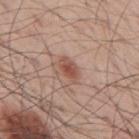The lesion is on the back.
The lesion-visualizer software estimated a mean CIELAB color near L≈52 a*≈22 b*≈28, roughly 11 lightness units darker than nearby skin, and a normalized lesion–skin contrast near 8.
The patient is a male aged 53–57.
Cropped from a total-body skin-imaging series; the visible field is about 15 mm.
This is a white-light tile.